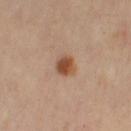follow-up = no biopsy performed (imaged during a skin exam) | TBP lesion metrics = an area of roughly 5 mm², a shape eccentricity near 0.4, and two-axis asymmetry of about 0.2; a lesion color around L≈50 a*≈22 b*≈33 in CIELAB and a normalized border contrast of about 10; border irregularity of about 1.5 on a 0–10 scale and internal color variation of about 4.5 on a 0–10 scale; a classifier nevus-likeness of about 100/100 and a lesion-detection confidence of about 100/100 | lesion diameter = about 2.5 mm | location = the leg | acquisition = 15 mm crop, total-body photography | illumination = cross-polarized | patient = female, about 40 years old.